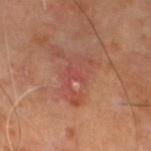Clinical impression: Captured during whole-body skin photography for melanoma surveillance; the lesion was not biopsied.